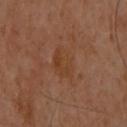- notes: no biopsy performed (imaged during a skin exam)
- image source: total-body-photography crop, ~15 mm field of view
- size: ≈3.5 mm
- site: the left arm
- lighting: cross-polarized illumination
- subject: female, aged approximately 60